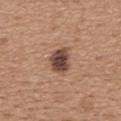The lesion was tiled from a total-body skin photograph and was not biopsied.
Located on the upper back.
The subject is a female aged approximately 45.
A region of skin cropped from a whole-body photographic capture, roughly 15 mm wide.
This is a white-light tile.
Measured at roughly 3.5 mm in maximum diameter.